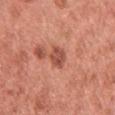Imaged during a routine full-body skin examination; the lesion was not biopsied and no histopathology is available. Located on the left upper arm. The subject is a female aged 48–52. Cropped from a total-body skin-imaging series; the visible field is about 15 mm. Imaged with white-light lighting. An algorithmic analysis of the crop reported a lesion-detection confidence of about 100/100. The lesion's longest dimension is about 3 mm.